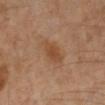biopsy status: no biopsy performed (imaged during a skin exam) | tile lighting: cross-polarized illumination | diameter: about 4 mm | acquisition: ~15 mm crop, total-body skin-cancer survey | site: the left lower leg | subject: male, approximately 55 years of age | automated metrics: a shape eccentricity near 0.85 and two-axis asymmetry of about 0.25; border irregularity of about 2.5 on a 0–10 scale and a color-variation rating of about 1.5/10.